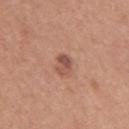{
  "biopsy_status": "not biopsied; imaged during a skin examination",
  "automated_metrics": {
    "area_mm2_approx": 4.0,
    "eccentricity": 0.8,
    "cielab_L": 52,
    "cielab_a": 23,
    "cielab_b": 28,
    "vs_skin_darker_L": 11.0,
    "vs_skin_contrast_norm": 7.5,
    "nevus_likeness_0_100": 50,
    "lesion_detection_confidence_0_100": 100
  },
  "site": "arm",
  "lighting": "white-light",
  "patient": {
    "sex": "female",
    "age_approx": 30
  },
  "image": {
    "source": "total-body photography crop",
    "field_of_view_mm": 15
  },
  "lesion_size": {
    "long_diameter_mm_approx": 3.0
  }
}